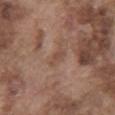Case summary:
– workup · imaged on a skin check; not biopsied
– lighting · white-light illumination
– subject · male, about 75 years old
– TBP lesion metrics · a lesion area of about 3 mm², an outline eccentricity of about 0.85 (0 = round, 1 = elongated), and two-axis asymmetry of about 0.3; a within-lesion color-variation index near 0.5/10
– anatomic site · the abdomen
– imaging modality · ~15 mm tile from a whole-body skin photo